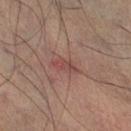Case summary:
• workup · total-body-photography surveillance lesion; no biopsy
• site · the left leg
• lighting · cross-polarized
• acquisition · ~15 mm crop, total-body skin-cancer survey
• patient · male, about 50 years old
• size · ~3 mm (longest diameter)